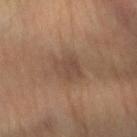Assessment: The lesion was tiled from a total-body skin photograph and was not biopsied. Background: An algorithmic analysis of the crop reported roughly 6 lightness units darker than nearby skin and a lesion-to-skin contrast of about 5.5 (normalized; higher = more distinct). From the arm. Longest diameter approximately 3 mm. A close-up tile cropped from a whole-body skin photograph, about 15 mm across. A male subject aged approximately 65. Imaged with cross-polarized lighting.